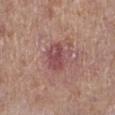  biopsy_status: not biopsied; imaged during a skin examination
  site: left leg
  patient:
    sex: male
    age_approx: 80
  image:
    source: total-body photography crop
    field_of_view_mm: 15